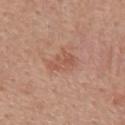| field | value |
|---|---|
| workup | no biopsy performed (imaged during a skin exam) |
| patient | male, aged approximately 55 |
| image source | ~15 mm crop, total-body skin-cancer survey |
| lesion diameter | about 3.5 mm |
| anatomic site | the mid back |
| tile lighting | white-light illumination |
| automated lesion analysis | a lesion area of about 5.5 mm², a shape eccentricity near 0.8, and a shape-asymmetry score of about 0.3 (0 = symmetric); a normalized lesion–skin contrast near 5; internal color variation of about 2.5 on a 0–10 scale and a peripheral color-asymmetry measure near 1; a nevus-likeness score of about 5/100 and lesion-presence confidence of about 100/100 |